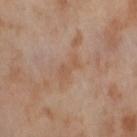The lesion was tiled from a total-body skin photograph and was not biopsied. The lesion is located on the right thigh. Measured at roughly 3 mm in maximum diameter. A female subject, roughly 55 years of age. This image is a 15 mm lesion crop taken from a total-body photograph.